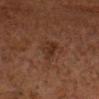  biopsy_status: not biopsied; imaged during a skin examination
  site: head or neck
  lighting: cross-polarized
  patient:
    sex: male
    age_approx: 65
  image:
    source: total-body photography crop
    field_of_view_mm: 15
  automated_metrics:
    eccentricity: 0.8
    cielab_L: 24
    cielab_a: 17
    cielab_b: 23
    vs_skin_contrast_norm: 6.5
    nevus_likeness_0_100: 15
    lesion_detection_confidence_0_100: 100
  lesion_size:
    long_diameter_mm_approx: 3.5